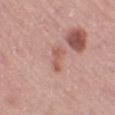No biopsy was performed on this lesion — it was imaged during a full skin examination and was not determined to be concerning. The total-body-photography lesion software estimated a mean CIELAB color near L≈57 a*≈24 b*≈27 and a lesion–skin lightness drop of about 8. The software also gave an automated nevus-likeness rating near 0 out of 100 and a lesion-detection confidence of about 100/100. Located on the left thigh. A female subject aged around 50. Imaged with white-light lighting. About 3 mm across. Cropped from a whole-body photographic skin survey; the tile spans about 15 mm.